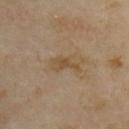Q: What is the imaging modality?
A: 15 mm crop, total-body photography
Q: Who is the patient?
A: female, approximately 35 years of age
Q: Where on the body is the lesion?
A: the upper back